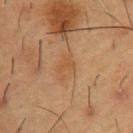Imaged during a routine full-body skin examination; the lesion was not biopsied and no histopathology is available.
The recorded lesion diameter is about 3 mm.
Cropped from a whole-body photographic skin survey; the tile spans about 15 mm.
Automated image analysis of the tile measured a footprint of about 3.5 mm², an eccentricity of roughly 0.8, and two-axis asymmetry of about 0.55. The analysis additionally found a border-irregularity rating of about 6.5/10, a within-lesion color-variation index near 0.5/10, and radial color variation of about 0. The analysis additionally found a classifier nevus-likeness of about 0/100 and a detector confidence of about 100 out of 100 that the crop contains a lesion.
From the chest.
The patient is a male aged approximately 55.
Captured under cross-polarized illumination.